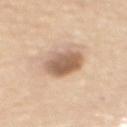- follow-up — imaged on a skin check; not biopsied
- image — ~15 mm crop, total-body skin-cancer survey
- image-analysis metrics — a footprint of about 12 mm², an outline eccentricity of about 0.45 (0 = round, 1 = elongated), and a symmetry-axis asymmetry near 0.15; a mean CIELAB color near L≈60 a*≈18 b*≈30, roughly 15 lightness units darker than nearby skin, and a normalized border contrast of about 9; a border-irregularity rating of about 1.5/10, internal color variation of about 5 on a 0–10 scale, and peripheral color asymmetry of about 1.5; lesion-presence confidence of about 100/100
- subject — female, aged around 65
- size — ~4 mm (longest diameter)
- site — the mid back